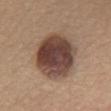{"biopsy_status": "not biopsied; imaged during a skin examination", "image": {"source": "total-body photography crop", "field_of_view_mm": 15}, "patient": {"sex": "female", "age_approx": 60}, "site": "chest"}